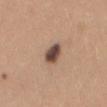Impression: This lesion was catalogued during total-body skin photography and was not selected for biopsy. Context: From the lower back. A close-up tile cropped from a whole-body skin photograph, about 15 mm across. Automated image analysis of the tile measured an area of roughly 5 mm², an eccentricity of roughly 0.75, and a shape-asymmetry score of about 0.15 (0 = symmetric). And it measured a border-irregularity rating of about 1.5/10, a within-lesion color-variation index near 5.5/10, and a peripheral color-asymmetry measure near 1.5. The software also gave an automated nevus-likeness rating near 70 out of 100 and a detector confidence of about 100 out of 100 that the crop contains a lesion. The lesion's longest dimension is about 3 mm. A female patient about 45 years old.